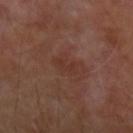Recorded during total-body skin imaging; not selected for excision or biopsy. A male subject aged approximately 50. A 15 mm close-up tile from a total-body photography series done for melanoma screening. From the left forearm. This is a cross-polarized tile. Longest diameter approximately 2.5 mm.